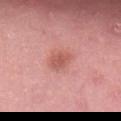The lesion was tiled from a total-body skin photograph and was not biopsied. The subject is a female about 50 years old. A 15 mm crop from a total-body photograph taken for skin-cancer surveillance. Located on the back. The tile uses white-light illumination.